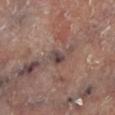<lesion>
<biopsy_status>not biopsied; imaged during a skin examination</biopsy_status>
<site>left lower leg</site>
<image>
  <source>total-body photography crop</source>
  <field_of_view_mm>15</field_of_view_mm>
</image>
<lighting>cross-polarized</lighting>
<automated_metrics>
  <cielab_L>43</cielab_L>
  <cielab_a>16</cielab_a>
  <cielab_b>18</cielab_b>
  <vs_skin_darker_L>9.0</vs_skin_darker_L>
  <vs_skin_contrast_norm>8.0</vs_skin_contrast_norm>
</automated_metrics>
<lesion_size>
  <long_diameter_mm_approx>2.5</long_diameter_mm_approx>
</lesion_size>
</lesion>